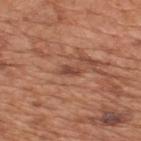Part of a total-body skin-imaging series; this lesion was reviewed on a skin check and was not flagged for biopsy.
The subject is a male approximately 65 years of age.
From the upper back.
Approximately 2.5 mm at its widest.
The lesion-visualizer software estimated a footprint of about 2.5 mm², an outline eccentricity of about 0.9 (0 = round, 1 = elongated), and two-axis asymmetry of about 0.45. The analysis additionally found a mean CIELAB color near L≈46 a*≈24 b*≈30, roughly 10 lightness units darker than nearby skin, and a normalized lesion–skin contrast near 7.5. The software also gave lesion-presence confidence of about 85/100.
This is a white-light tile.
A close-up tile cropped from a whole-body skin photograph, about 15 mm across.